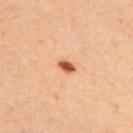imaging modality=~15 mm tile from a whole-body skin photo; patient=male, approximately 45 years of age; location=the upper back.